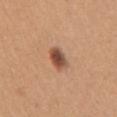<record>
  <biopsy_status>not biopsied; imaged during a skin examination</biopsy_status>
  <lesion_size>
    <long_diameter_mm_approx>3.0</long_diameter_mm_approx>
  </lesion_size>
  <patient>
    <sex>female</sex>
    <age_approx>30</age_approx>
  </patient>
  <site>right upper arm</site>
  <lighting>white-light</lighting>
  <image>
    <source>total-body photography crop</source>
    <field_of_view_mm>15</field_of_view_mm>
  </image>
</record>